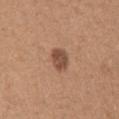Assessment:
This lesion was catalogued during total-body skin photography and was not selected for biopsy.
Clinical summary:
A female patient approximately 45 years of age. A 15 mm close-up extracted from a 3D total-body photography capture. Captured under white-light illumination. The lesion is located on the right upper arm. Longest diameter approximately 3 mm. Automated tile analysis of the lesion measured a footprint of about 5.5 mm² and an eccentricity of roughly 0.6. The analysis additionally found a lesion color around L≈49 a*≈21 b*≈29 in CIELAB, a lesion–skin lightness drop of about 12, and a normalized lesion–skin contrast near 8.5. The analysis additionally found an automated nevus-likeness rating near 70 out of 100 and lesion-presence confidence of about 100/100.